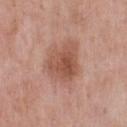Part of a total-body skin-imaging series; this lesion was reviewed on a skin check and was not flagged for biopsy. A male subject about 55 years old. Measured at roughly 4.5 mm in maximum diameter. The lesion is located on the chest. A 15 mm close-up tile from a total-body photography series done for melanoma screening. Imaged with white-light lighting.